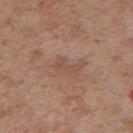notes: total-body-photography surveillance lesion; no biopsy | image source: ~15 mm crop, total-body skin-cancer survey | size: ≈3 mm | subject: male, aged 48 to 52 | anatomic site: the right upper arm | tile lighting: white-light illumination.